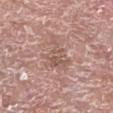notes: total-body-photography surveillance lesion; no biopsy
lighting: white-light
image: ~15 mm crop, total-body skin-cancer survey
automated metrics: an area of roughly 9.5 mm², a shape eccentricity near 0.65, and a symmetry-axis asymmetry near 0.55; an average lesion color of about L≈56 a*≈19 b*≈24 (CIELAB) and a normalized lesion–skin contrast near 5; a border-irregularity index near 7/10, a within-lesion color-variation index near 3.5/10, and radial color variation of about 1.5; a classifier nevus-likeness of about 0/100 and lesion-presence confidence of about 95/100
body site: the right lower leg
subject: male, aged approximately 75
diameter: ≈5 mm